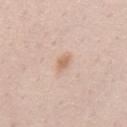This lesion was catalogued during total-body skin photography and was not selected for biopsy.
From the mid back.
Imaged with white-light lighting.
A male patient, approximately 35 years of age.
A region of skin cropped from a whole-body photographic capture, roughly 15 mm wide.